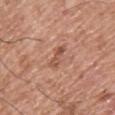This lesion was catalogued during total-body skin photography and was not selected for biopsy.
About 3.5 mm across.
A male subject, in their mid- to late 60s.
Cropped from a whole-body photographic skin survey; the tile spans about 15 mm.
The lesion is located on the back.
The total-body-photography lesion software estimated a lesion area of about 4 mm², an outline eccentricity of about 0.95 (0 = round, 1 = elongated), and two-axis asymmetry of about 0.35.
The tile uses white-light illumination.